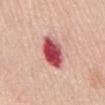  patient:
    sex: female
    age_approx: 65
  lesion_size:
    long_diameter_mm_approx: 5.0
  automated_metrics:
    area_mm2_approx: 11.0
    eccentricity: 0.8
    shape_asymmetry: 0.15
    cielab_L: 53
    cielab_a: 37
    cielab_b: 23
    vs_skin_darker_L: 21.0
    vs_skin_contrast_norm: 13.0
    border_irregularity_0_10: 1.5
    color_variation_0_10: 10.0
    peripheral_color_asymmetry: 3.5
    nevus_likeness_0_100: 0
    lesion_detection_confidence_0_100: 100
  lighting: white-light
  image:
    source: total-body photography crop
    field_of_view_mm: 15
  site: chest